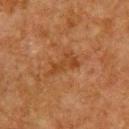notes — imaged on a skin check; not biopsied | illumination — cross-polarized illumination | anatomic site — the upper back | lesion size — about 4 mm | patient — male, in their mid-60s | image source — ~15 mm tile from a whole-body skin photo.